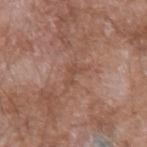Captured during whole-body skin photography for melanoma surveillance; the lesion was not biopsied.
A 15 mm close-up tile from a total-body photography series done for melanoma screening.
The lesion is on the left forearm.
This is a white-light tile.
Measured at roughly 2.5 mm in maximum diameter.
A male subject approximately 60 years of age.